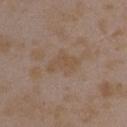notes: no biopsy performed (imaged during a skin exam); image-analysis metrics: a border-irregularity rating of about 5.5/10, a color-variation rating of about 1/10, and peripheral color asymmetry of about 0; site: the right upper arm; subject: female, aged around 35; lesion size: ≈3.5 mm; illumination: white-light illumination; image source: total-body-photography crop, ~15 mm field of view.